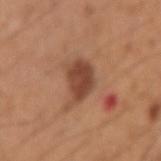Q: Was this lesion biopsied?
A: total-body-photography surveillance lesion; no biopsy
Q: What lighting was used for the tile?
A: white-light illumination
Q: How was this image acquired?
A: ~15 mm tile from a whole-body skin photo
Q: Who is the patient?
A: male, aged approximately 35
Q: What is the anatomic site?
A: the right upper arm
Q: How large is the lesion?
A: ≈4 mm